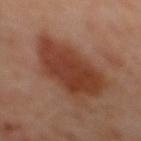A male subject, aged 58–62.
The tile uses cross-polarized illumination.
Located on the mid back.
The total-body-photography lesion software estimated a shape eccentricity near 0.85 and a symmetry-axis asymmetry near 0.25. And it measured a normalized lesion–skin contrast near 9.5. The analysis additionally found a border-irregularity rating of about 3.5/10, a color-variation rating of about 3.5/10, and radial color variation of about 1.
The recorded lesion diameter is about 8.5 mm.
Cropped from a total-body skin-imaging series; the visible field is about 15 mm.